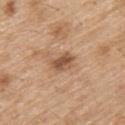Clinical impression: Imaged during a routine full-body skin examination; the lesion was not biopsied and no histopathology is available. Clinical summary: Located on the upper back. Captured under white-light illumination. Approximately 3 mm at its widest. The patient is a male aged 68 to 72. Automated tile analysis of the lesion measured a mean CIELAB color near L≈53 a*≈20 b*≈33, about 12 CIELAB-L* units darker than the surrounding skin, and a normalized border contrast of about 8. The analysis additionally found a border-irregularity rating of about 2.5/10 and internal color variation of about 4 on a 0–10 scale. Cropped from a whole-body photographic skin survey; the tile spans about 15 mm.